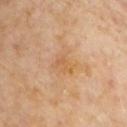notes — catalogued during a skin exam; not biopsied
image source — total-body-photography crop, ~15 mm field of view
site — the chest
patient — male, roughly 55 years of age
tile lighting — cross-polarized illumination
diameter — ~3.5 mm (longest diameter)